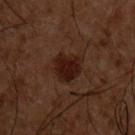Q: Is there a histopathology result?
A: total-body-photography surveillance lesion; no biopsy
Q: How was this image acquired?
A: ~15 mm crop, total-body skin-cancer survey
Q: Automated lesion metrics?
A: a lesion area of about 8.5 mm² and a shape eccentricity near 0.7; a normalized border contrast of about 10; border irregularity of about 2.5 on a 0–10 scale, a color-variation rating of about 2/10, and radial color variation of about 0.5; a classifier nevus-likeness of about 90/100 and a lesion-detection confidence of about 100/100
Q: Lesion size?
A: about 4 mm
Q: Who is the patient?
A: male, roughly 50 years of age
Q: Where on the body is the lesion?
A: the chest
Q: Illumination type?
A: cross-polarized